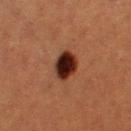{
  "biopsy_status": "not biopsied; imaged during a skin examination",
  "site": "left thigh",
  "image": {
    "source": "total-body photography crop",
    "field_of_view_mm": 15
  },
  "patient": {
    "sex": "female",
    "age_approx": 40
  },
  "lesion_size": {
    "long_diameter_mm_approx": 3.5
  }
}